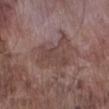| field | value |
|---|---|
| follow-up | catalogued during a skin exam; not biopsied |
| patient | male, in their mid- to late 70s |
| image source | ~15 mm crop, total-body skin-cancer survey |
| anatomic site | the right lower leg |
| diameter | about 5.5 mm |